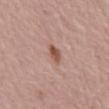Captured during whole-body skin photography for melanoma surveillance; the lesion was not biopsied.